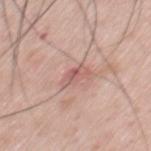Assessment: Imaged during a routine full-body skin examination; the lesion was not biopsied and no histopathology is available. Background: From the front of the torso. The subject is a male in their 60s. A close-up tile cropped from a whole-body skin photograph, about 15 mm across.